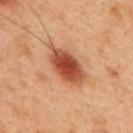Located on the upper back.
This is a cross-polarized tile.
The subject is a male aged 48–52.
A close-up tile cropped from a whole-body skin photograph, about 15 mm across.
Measured at roughly 5.5 mm in maximum diameter.
An algorithmic analysis of the crop reported a lesion area of about 13 mm², an outline eccentricity of about 0.85 (0 = round, 1 = elongated), and a shape-asymmetry score of about 0.2 (0 = symmetric). And it measured border irregularity of about 2 on a 0–10 scale, a color-variation rating of about 5.5/10, and a peripheral color-asymmetry measure near 1. The analysis additionally found lesion-presence confidence of about 100/100.